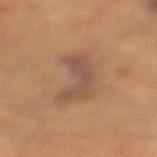Clinical impression: Imaged during a routine full-body skin examination; the lesion was not biopsied and no histopathology is available. Clinical summary: On the left lower leg. This is a cross-polarized tile. Cropped from a whole-body photographic skin survey; the tile spans about 15 mm. A male subject aged approximately 85 or older.